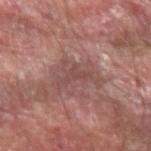Q: What are the patient's age and sex?
A: male, roughly 55 years of age
Q: What is the imaging modality?
A: 15 mm crop, total-body photography
Q: Where on the body is the lesion?
A: the arm
Q: Lesion size?
A: about 4 mm